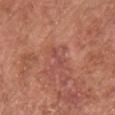Part of a total-body skin-imaging series; this lesion was reviewed on a skin check and was not flagged for biopsy. On the chest. A male patient, in their mid-70s. Cropped from a whole-body photographic skin survey; the tile spans about 15 mm.